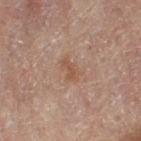biopsy status: catalogued during a skin exam; not biopsied
image source: ~15 mm tile from a whole-body skin photo
subject: female, in their 80s
tile lighting: cross-polarized illumination
anatomic site: the right leg
size: ~3 mm (longest diameter)